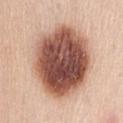The lesion was photographed on a routine skin check and not biopsied; there is no pathology result. Cropped from a total-body skin-imaging series; the visible field is about 15 mm. Measured at roughly 9 mm in maximum diameter. From the abdomen. The patient is a female approximately 55 years of age. The tile uses white-light illumination.